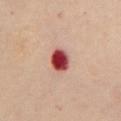No biopsy was performed on this lesion — it was imaged during a full skin examination and was not determined to be concerning. A 15 mm crop from a total-body photograph taken for skin-cancer surveillance. Captured under cross-polarized illumination. The lesion-visualizer software estimated an average lesion color of about L≈46 a*≈36 b*≈27 (CIELAB). The analysis additionally found a border-irregularity index near 1.5/10 and a color-variation rating of about 6.5/10. And it measured a nevus-likeness score of about 0/100 and a lesion-detection confidence of about 100/100. A female subject approximately 60 years of age. Located on the chest.